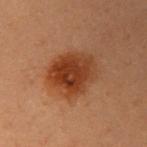Assessment: This lesion was catalogued during total-body skin photography and was not selected for biopsy. Context: This image is a 15 mm lesion crop taken from a total-body photograph. Located on the right upper arm. The lesion's longest dimension is about 5.5 mm. A female patient, aged 38 to 42. This is a cross-polarized tile.